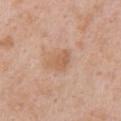  biopsy_status: not biopsied; imaged during a skin examination
  automated_metrics:
    cielab_L: 60
    cielab_a: 20
    cielab_b: 33
    vs_skin_darker_L: 8.0
    border_irregularity_0_10: 3.5
    color_variation_0_10: 2.0
    peripheral_color_asymmetry: 0.5
  patient:
    sex: female
    age_approx: 40
  site: chest
  lighting: white-light
  lesion_size:
    long_diameter_mm_approx: 3.5
  image:
    source: total-body photography crop
    field_of_view_mm: 15